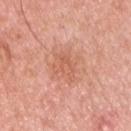follow-up — imaged on a skin check; not biopsied
automated metrics — border irregularity of about 4 on a 0–10 scale and radial color variation of about 1
diameter — about 4 mm
image source — ~15 mm crop, total-body skin-cancer survey
patient — male, aged 43–47
tile lighting — white-light illumination
location — the chest